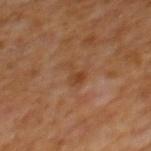This lesion was catalogued during total-body skin photography and was not selected for biopsy.